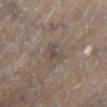The lesion was photographed on a routine skin check and not biopsied; there is no pathology result. On the leg. Captured under cross-polarized illumination. A male subject aged around 85 or older. Longest diameter approximately 3 mm. Cropped from a whole-body photographic skin survey; the tile spans about 15 mm.